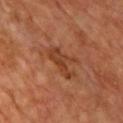workup=catalogued during a skin exam; not biopsied
image-analysis metrics=a border-irregularity rating of about 7.5/10 and radial color variation of about 0.5
site=the chest
tile lighting=cross-polarized illumination
lesion diameter=≈4.5 mm
acquisition=15 mm crop, total-body photography
subject=male, aged 63 to 67